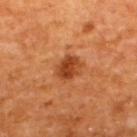biopsy status — catalogued during a skin exam; not biopsied | anatomic site — the upper back | subject — male, about 65 years old | image source — 15 mm crop, total-body photography.